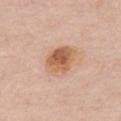Recorded during total-body skin imaging; not selected for excision or biopsy. A roughly 15 mm field-of-view crop from a total-body skin photograph. The tile uses white-light illumination. A male patient, approximately 75 years of age. From the chest. An algorithmic analysis of the crop reported an average lesion color of about L≈61 a*≈21 b*≈34 (CIELAB), roughly 11 lightness units darker than nearby skin, and a normalized lesion–skin contrast near 8.5.